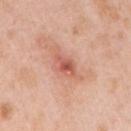Automated tile analysis of the lesion measured a mean CIELAB color near L≈57 a*≈29 b*≈31 and roughly 11 lightness units darker than nearby skin. It also reported a border-irregularity rating of about 3.5/10 and peripheral color asymmetry of about 1.
A close-up tile cropped from a whole-body skin photograph, about 15 mm across.
The patient is a female aged 43 to 47.
About 3 mm across.
The lesion is on the right upper arm.